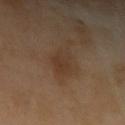biopsy_status: not biopsied; imaged during a skin examination
site: left upper arm
patient:
  sex: male
  age_approx: 70
image:
  source: total-body photography crop
  field_of_view_mm: 15